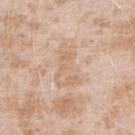<case>
<image>
  <source>total-body photography crop</source>
  <field_of_view_mm>15</field_of_view_mm>
</image>
<patient>
  <sex>female</sex>
  <age_approx>25</age_approx>
</patient>
<site>right forearm</site>
<lesion_size>
  <long_diameter_mm_approx>5.0</long_diameter_mm_approx>
</lesion_size>
</case>